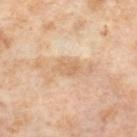Imaged during a routine full-body skin examination; the lesion was not biopsied and no histopathology is available.
A 15 mm close-up tile from a total-body photography series done for melanoma screening.
The lesion is on the right thigh.
The subject is a female aged 53–57.
The lesion's longest dimension is about 3 mm.